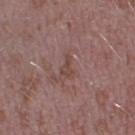biopsy_status: not biopsied; imaged during a skin examination
lesion_size:
  long_diameter_mm_approx: 2.5
site: left lower leg
image:
  source: total-body photography crop
  field_of_view_mm: 15
patient:
  sex: male
  age_approx: 40